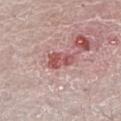Q: Is there a histopathology result?
A: no biopsy performed (imaged during a skin exam)
Q: How was this image acquired?
A: total-body-photography crop, ~15 mm field of view
Q: How large is the lesion?
A: ≈3.5 mm
Q: Lesion location?
A: the left lower leg
Q: How was the tile lit?
A: white-light
Q: What are the patient's age and sex?
A: female, in their mid-50s